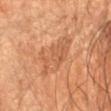| feature | finding |
|---|---|
| biopsy status | catalogued during a skin exam; not biopsied |
| subject | male, approximately 55 years of age |
| body site | the left forearm |
| illumination | cross-polarized illumination |
| imaging modality | 15 mm crop, total-body photography |